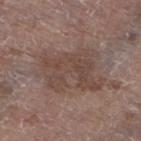A female patient, aged 83 or older. The recorded lesion diameter is about 7.5 mm. The lesion is on the left lower leg. This image is a 15 mm lesion crop taken from a total-body photograph. Imaged with white-light lighting.